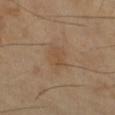Q: Was this lesion biopsied?
A: imaged on a skin check; not biopsied
Q: What are the patient's age and sex?
A: female, approximately 70 years of age
Q: Where on the body is the lesion?
A: the leg
Q: How was this image acquired?
A: ~15 mm tile from a whole-body skin photo
Q: What is the lesion's diameter?
A: ≈3.5 mm